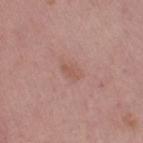workup: no biopsy performed (imaged during a skin exam) | patient: female, aged around 65 | anatomic site: the left thigh | acquisition: 15 mm crop, total-body photography.